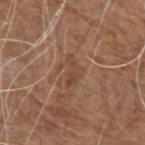Impression:
Imaged during a routine full-body skin examination; the lesion was not biopsied and no histopathology is available.
Background:
The lesion is located on the left upper arm. Automated image analysis of the tile measured a lesion area of about 3.5 mm² and a shape eccentricity near 0.9. And it measured an average lesion color of about L≈44 a*≈20 b*≈28 (CIELAB) and roughly 7 lightness units darker than nearby skin. The software also gave a border-irregularity rating of about 7/10. Longest diameter approximately 3.5 mm. A 15 mm crop from a total-body photograph taken for skin-cancer surveillance. Imaged with white-light lighting. The subject is a male in their 80s.